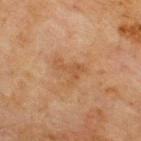Case summary:
- location · the upper back
- image · total-body-photography crop, ~15 mm field of view
- subject · male, approximately 70 years of age